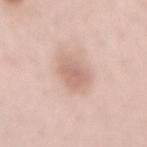biopsy status = no biopsy performed (imaged during a skin exam)
tile lighting = white-light
lesion diameter = ~4.5 mm (longest diameter)
image source = ~15 mm crop, total-body skin-cancer survey
patient = female, aged approximately 50
location = the mid back